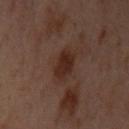Imaged during a routine full-body skin examination; the lesion was not biopsied and no histopathology is available.